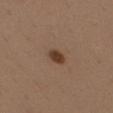Clinical impression:
Imaged during a routine full-body skin examination; the lesion was not biopsied and no histopathology is available.
Image and clinical context:
A close-up tile cropped from a whole-body skin photograph, about 15 mm across. On the mid back. Measured at roughly 2.5 mm in maximum diameter. A male patient, about 40 years old.